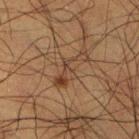Clinical impression:
Recorded during total-body skin imaging; not selected for excision or biopsy.
Background:
A lesion tile, about 15 mm wide, cut from a 3D total-body photograph. The lesion is located on the right lower leg. A male subject about 50 years old.